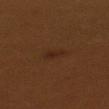biopsy_status: not biopsied; imaged during a skin examination
automated_metrics:
  cielab_L: 22
  cielab_a: 17
  cielab_b: 24
  vs_skin_darker_L: 4.0
image:
  source: total-body photography crop
  field_of_view_mm: 15
site: upper back
lesion_size:
  long_diameter_mm_approx: 2.5
lighting: cross-polarized
patient:
  sex: female
  age_approx: 30